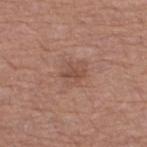Q: Is there a histopathology result?
A: catalogued during a skin exam; not biopsied
Q: What is the imaging modality?
A: ~15 mm crop, total-body skin-cancer survey
Q: Where on the body is the lesion?
A: the right thigh
Q: What lighting was used for the tile?
A: white-light illumination
Q: What did automated image analysis measure?
A: a lesion area of about 2.5 mm², an outline eccentricity of about 0.9 (0 = round, 1 = elongated), and a symmetry-axis asymmetry near 0.55; a lesion color around L≈48 a*≈21 b*≈27 in CIELAB and a normalized lesion–skin contrast near 6.5; a border-irregularity index near 5.5/10, a within-lesion color-variation index near 0/10, and peripheral color asymmetry of about 0
Q: How large is the lesion?
A: ≈2.5 mm
Q: What are the patient's age and sex?
A: female, approximately 50 years of age